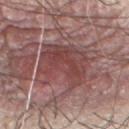This lesion was catalogued during total-body skin photography and was not selected for biopsy.
From the chest.
A male subject, aged 73–77.
A 15 mm crop from a total-body photograph taken for skin-cancer surveillance.